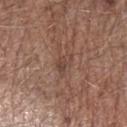Findings:
• workup: total-body-photography surveillance lesion; no biopsy
• patient: male, aged around 70
• lesion diameter: ≈2.5 mm
• illumination: white-light
• image: total-body-photography crop, ~15 mm field of view
• body site: the left forearm